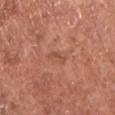Q: How was this image acquired?
A: ~15 mm tile from a whole-body skin photo
Q: Where on the body is the lesion?
A: the chest
Q: Who is the patient?
A: male, in their mid-70s
Q: How large is the lesion?
A: ~2.5 mm (longest diameter)